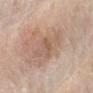workup: no biopsy performed (imaged during a skin exam)
image-analysis metrics: an area of roughly 13 mm², an eccentricity of roughly 0.9, and a shape-asymmetry score of about 0.3 (0 = symmetric); a mean CIELAB color near L≈59 a*≈17 b*≈28, a lesion–skin lightness drop of about 9, and a normalized lesion–skin contrast near 6; border irregularity of about 4 on a 0–10 scale, a color-variation rating of about 5.5/10, and peripheral color asymmetry of about 2; a classifier nevus-likeness of about 0/100 and a detector confidence of about 100 out of 100 that the crop contains a lesion
anatomic site: the arm
size: about 6 mm
image: total-body-photography crop, ~15 mm field of view
subject: female, about 70 years old